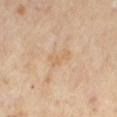Imaged during a routine full-body skin examination; the lesion was not biopsied and no histopathology is available.
Captured under cross-polarized illumination.
The total-body-photography lesion software estimated an average lesion color of about L≈64 a*≈16 b*≈35 (CIELAB), a lesion–skin lightness drop of about 6, and a normalized lesion–skin contrast near 4.5.
On the mid back.
A female subject, about 60 years old.
A roughly 15 mm field-of-view crop from a total-body skin photograph.